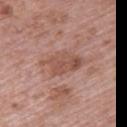workup: no biopsy performed (imaged during a skin exam) | tile lighting: white-light illumination | body site: the upper back | image source: total-body-photography crop, ~15 mm field of view | subject: female, in their 60s.